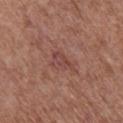Assessment:
This lesion was catalogued during total-body skin photography and was not selected for biopsy.
Clinical summary:
A roughly 15 mm field-of-view crop from a total-body skin photograph. The total-body-photography lesion software estimated a lesion area of about 4.5 mm², an outline eccentricity of about 0.85 (0 = round, 1 = elongated), and two-axis asymmetry of about 0.45. The lesion is located on the arm. A female patient, in their mid- to late 60s. The recorded lesion diameter is about 3.5 mm. Captured under white-light illumination.